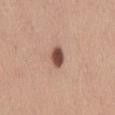Clinical impression: No biopsy was performed on this lesion — it was imaged during a full skin examination and was not determined to be concerning. Image and clinical context: On the abdomen. Cropped from a total-body skin-imaging series; the visible field is about 15 mm. The patient is a male approximately 30 years of age. The total-body-photography lesion software estimated a lesion area of about 4.5 mm², an eccentricity of roughly 0.75, and a shape-asymmetry score of about 0.3 (0 = symmetric). The analysis additionally found a within-lesion color-variation index near 3/10 and peripheral color asymmetry of about 1. The software also gave a nevus-likeness score of about 100/100 and a lesion-detection confidence of about 100/100. About 2.5 mm across.